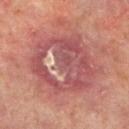Case summary:
* anatomic site — the left lower leg
* subject — male, aged approximately 65
* imaging modality — total-body-photography crop, ~15 mm field of view
* TBP lesion metrics — a border-irregularity index near 2.5/10, internal color variation of about 9.5 on a 0–10 scale, and a peripheral color-asymmetry measure near 3; a classifier nevus-likeness of about 0/100 and a lesion-detection confidence of about 55/100